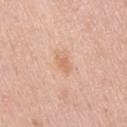| field | value |
|---|---|
| biopsy status | total-body-photography surveillance lesion; no biopsy |
| imaging modality | total-body-photography crop, ~15 mm field of view |
| body site | the right upper arm |
| patient | male, roughly 45 years of age |
| tile lighting | white-light illumination |
| lesion size | ≈2.5 mm |
| automated metrics | a mean CIELAB color near L≈66 a*≈23 b*≈33, about 8 CIELAB-L* units darker than the surrounding skin, and a lesion-to-skin contrast of about 5.5 (normalized; higher = more distinct); a classifier nevus-likeness of about 0/100 |